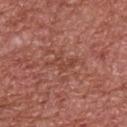image source = ~15 mm crop, total-body skin-cancer survey
illumination = white-light illumination
subject = male, aged 63–67
lesion diameter = about 2.5 mm
location = the upper back
image-analysis metrics = an area of roughly 3.5 mm², an eccentricity of roughly 0.8, and two-axis asymmetry of about 0.65; a mean CIELAB color near L≈45 a*≈26 b*≈29 and a lesion–skin lightness drop of about 6